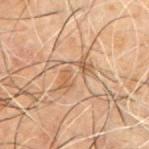Notes:
– workup — imaged on a skin check; not biopsied
– diameter — ~4.5 mm (longest diameter)
– automated lesion analysis — a footprint of about 8.5 mm² and an eccentricity of roughly 0.85; a border-irregularity rating of about 5/10; an automated nevus-likeness rating near 0 out of 100 and a lesion-detection confidence of about 90/100
– image — 15 mm crop, total-body photography
– location — the chest
– patient — male, approximately 50 years of age
– illumination — cross-polarized